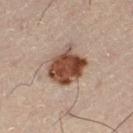The lesion is located on the left thigh.
A male patient, roughly 50 years of age.
Cropped from a total-body skin-imaging series; the visible field is about 15 mm.